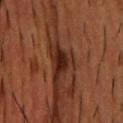The patient is a male approximately 55 years of age. The lesion is on the chest. Captured under cross-polarized illumination. A 15 mm crop from a total-body photograph taken for skin-cancer surveillance. An algorithmic analysis of the crop reported roughly 11 lightness units darker than nearby skin and a normalized lesion–skin contrast near 12.5. The software also gave a border-irregularity index near 3/10, a color-variation rating of about 2/10, and peripheral color asymmetry of about 1. The analysis additionally found a classifier nevus-likeness of about 20/100 and lesion-presence confidence of about 100/100. The recorded lesion diameter is about 3 mm.